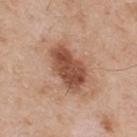Assessment:
Captured during whole-body skin photography for melanoma surveillance; the lesion was not biopsied.
Acquisition and patient details:
The lesion is on the upper back. Captured under white-light illumination. Automated tile analysis of the lesion measured a footprint of about 16 mm², a shape eccentricity near 0.85, and a symmetry-axis asymmetry near 0.25. The analysis additionally found a mean CIELAB color near L≈51 a*≈23 b*≈31, about 14 CIELAB-L* units darker than the surrounding skin, and a normalized lesion–skin contrast near 9.5. The software also gave a border-irregularity index near 2.5/10, a within-lesion color-variation index near 4.5/10, and a peripheral color-asymmetry measure near 1.5. A lesion tile, about 15 mm wide, cut from a 3D total-body photograph. Measured at roughly 6 mm in maximum diameter. A male patient aged approximately 55.